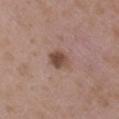Findings:
• notes: catalogued during a skin exam; not biopsied
• imaging modality: ~15 mm tile from a whole-body skin photo
• automated lesion analysis: a footprint of about 5 mm² and a shape eccentricity near 0.75; an average lesion color of about L≈47 a*≈18 b*≈25 (CIELAB), a lesion–skin lightness drop of about 12, and a normalized lesion–skin contrast near 9; a nevus-likeness score of about 90/100
• diameter: ≈3 mm
• subject: female, in their mid- to late 30s
• anatomic site: the right upper arm